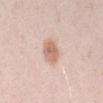No biopsy was performed on this lesion — it was imaged during a full skin examination and was not determined to be concerning. Cropped from a total-body skin-imaging series; the visible field is about 15 mm. The recorded lesion diameter is about 3 mm. The lesion is on the arm. A female subject, roughly 30 years of age. The tile uses white-light illumination.